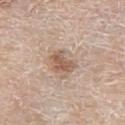The lesion was photographed on a routine skin check and not biopsied; there is no pathology result.
The total-body-photography lesion software estimated a lesion area of about 6 mm², an eccentricity of roughly 0.7, and two-axis asymmetry of about 0.35. The analysis additionally found a lesion color around L≈57 a*≈18 b*≈28 in CIELAB, about 11 CIELAB-L* units darker than the surrounding skin, and a lesion-to-skin contrast of about 7.5 (normalized; higher = more distinct). It also reported a border-irregularity index near 3.5/10, a color-variation rating of about 2/10, and peripheral color asymmetry of about 0.5.
Imaged with white-light lighting.
This image is a 15 mm lesion crop taken from a total-body photograph.
A male patient, approximately 80 years of age.
On the left thigh.
The lesion's longest dimension is about 3 mm.